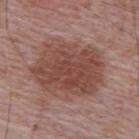• biopsy status · no biopsy performed (imaged during a skin exam)
• subject · male, aged 63–67
• acquisition · ~15 mm tile from a whole-body skin photo
• tile lighting · white-light
• lesion size · ≈8 mm
• body site · the upper back